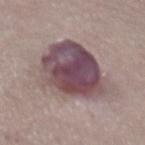{
  "biopsy_status": "not biopsied; imaged during a skin examination",
  "image": {
    "source": "total-body photography crop",
    "field_of_view_mm": 15
  },
  "lesion_size": {
    "long_diameter_mm_approx": 8.5
  },
  "automated_metrics": {
    "cielab_L": 46,
    "cielab_a": 19,
    "cielab_b": 12,
    "vs_skin_contrast_norm": 12.0,
    "lesion_detection_confidence_0_100": 55
  },
  "site": "abdomen",
  "patient": {
    "sex": "male",
    "age_approx": 75
  }
}